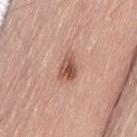| key | value |
|---|---|
| notes | catalogued during a skin exam; not biopsied |
| body site | the lower back |
| image source | ~15 mm crop, total-body skin-cancer survey |
| tile lighting | white-light |
| lesion size | ~3.5 mm (longest diameter) |
| subject | male, in their mid- to late 60s |